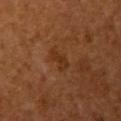Q: Is there a histopathology result?
A: imaged on a skin check; not biopsied
Q: What are the patient's age and sex?
A: female, about 65 years old
Q: How was this image acquired?
A: ~15 mm crop, total-body skin-cancer survey
Q: Where on the body is the lesion?
A: the left upper arm